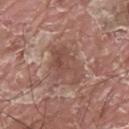{"biopsy_status": "not biopsied; imaged during a skin examination"}